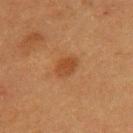The lesion was tiled from a total-body skin photograph and was not biopsied.
A female subject aged around 40.
About 3 mm across.
The lesion is on the left upper arm.
A 15 mm close-up tile from a total-body photography series done for melanoma screening.
This is a cross-polarized tile.